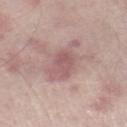biopsy status — total-body-photography surveillance lesion; no biopsy | location — the leg | subject — male, in their mid- to late 60s | illumination — white-light | diameter — ≈5 mm | image-analysis metrics — an area of roughly 11 mm², an outline eccentricity of about 0.65 (0 = round, 1 = elongated), and two-axis asymmetry of about 0.3; a normalized border contrast of about 6 | image — 15 mm crop, total-body photography.